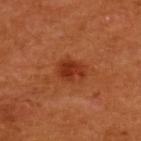The lesion was photographed on a routine skin check and not biopsied; there is no pathology result.
A female patient aged approximately 55.
The lesion is located on the back.
A close-up tile cropped from a whole-body skin photograph, about 15 mm across.
An algorithmic analysis of the crop reported a footprint of about 6.5 mm², a shape eccentricity near 0.7, and a symmetry-axis asymmetry near 0.3. The analysis additionally found an average lesion color of about L≈37 a*≈32 b*≈38 (CIELAB), about 10 CIELAB-L* units darker than the surrounding skin, and a normalized border contrast of about 8. The software also gave a border-irregularity index near 3/10, a within-lesion color-variation index near 3/10, and radial color variation of about 1. It also reported a nevus-likeness score of about 95/100 and lesion-presence confidence of about 100/100.